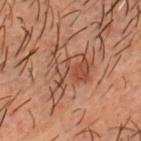Assessment:
The lesion was tiled from a total-body skin photograph and was not biopsied.
Background:
The tile uses cross-polarized illumination. From the head or neck. About 6 mm across. This image is a 15 mm lesion crop taken from a total-body photograph. The lesion-visualizer software estimated an area of roughly 15 mm², a shape eccentricity near 0.55, and two-axis asymmetry of about 0.65. The software also gave a mean CIELAB color near L≈40 a*≈19 b*≈26 and a lesion-to-skin contrast of about 6 (normalized; higher = more distinct). The analysis additionally found a border-irregularity rating of about 8.5/10, a within-lesion color-variation index near 6/10, and a peripheral color-asymmetry measure near 2. It also reported a classifier nevus-likeness of about 25/100 and a lesion-detection confidence of about 100/100. The patient is a male about 50 years old.